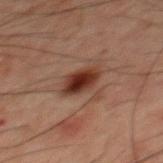biopsy_status: not biopsied; imaged during a skin examination
site: mid back
patient:
  sex: male
  age_approx: 60
automated_metrics:
  cielab_L: 25
  cielab_a: 19
  cielab_b: 22
  vs_skin_darker_L: 12.0
  vs_skin_contrast_norm: 12.0
  border_irregularity_0_10: 2.0
  color_variation_0_10: 4.5
  peripheral_color_asymmetry: 1.5
  lesion_detection_confidence_0_100: 100
lighting: cross-polarized
lesion_size:
  long_diameter_mm_approx: 3.5
image:
  source: total-body photography crop
  field_of_view_mm: 15